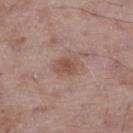<case>
<biopsy_status>not biopsied; imaged during a skin examination</biopsy_status>
<lesion_size>
  <long_diameter_mm_approx>3.0</long_diameter_mm_approx>
</lesion_size>
<site>leg</site>
<patient>
  <sex>male</sex>
  <age_approx>50</age_approx>
</patient>
<image>
  <source>total-body photography crop</source>
  <field_of_view_mm>15</field_of_view_mm>
</image>
</case>